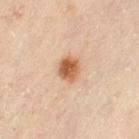Imaged during a routine full-body skin examination; the lesion was not biopsied and no histopathology is available.
Located on the left thigh.
The subject is a female about 60 years old.
A roughly 15 mm field-of-view crop from a total-body skin photograph.